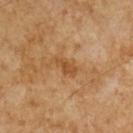notes: no biopsy performed (imaged during a skin exam).